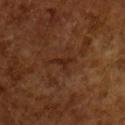<case>
  <biopsy_status>not biopsied; imaged during a skin examination</biopsy_status>
  <image>
    <source>total-body photography crop</source>
    <field_of_view_mm>15</field_of_view_mm>
  </image>
  <automated_metrics>
    <nevus_likeness_0_100>0</nevus_likeness_0_100>
    <lesion_detection_confidence_0_100>95</lesion_detection_confidence_0_100>
  </automated_metrics>
  <lesion_size>
    <long_diameter_mm_approx>3.0</long_diameter_mm_approx>
  </lesion_size>
  <patient>
    <sex>male</sex>
    <age_approx>65</age_approx>
  </patient>
  <lighting>cross-polarized</lighting>
</case>